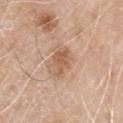Q: Was a biopsy performed?
A: no biopsy performed (imaged during a skin exam)
Q: What lighting was used for the tile?
A: white-light
Q: What are the patient's age and sex?
A: male, approximately 80 years of age
Q: What is the anatomic site?
A: the right upper arm
Q: What is the imaging modality?
A: total-body-photography crop, ~15 mm field of view
Q: Lesion size?
A: ≈3.5 mm